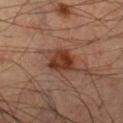<case>
  <biopsy_status>not biopsied; imaged during a skin examination</biopsy_status>
  <lighting>cross-polarized</lighting>
  <patient>
    <sex>male</sex>
    <age_approx>45</age_approx>
  </patient>
  <automated_metrics>
    <area_mm2_approx>9.5</area_mm2_approx>
    <eccentricity>0.8</eccentricity>
    <shape_asymmetry>0.2</shape_asymmetry>
  </automated_metrics>
  <site>right lower leg</site>
  <lesion_size>
    <long_diameter_mm_approx>5.0</long_diameter_mm_approx>
  </lesion_size>
  <image>
    <source>total-body photography crop</source>
    <field_of_view_mm>15</field_of_view_mm>
  </image>
</case>